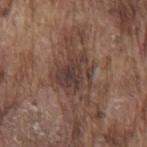Q: Is there a histopathology result?
A: catalogued during a skin exam; not biopsied
Q: Lesion location?
A: the mid back
Q: What did automated image analysis measure?
A: an outline eccentricity of about 0.5 (0 = round, 1 = elongated) and two-axis asymmetry of about 0.3; a nevus-likeness score of about 5/100 and a detector confidence of about 95 out of 100 that the crop contains a lesion
Q: Illumination type?
A: white-light
Q: What are the patient's age and sex?
A: male, approximately 75 years of age
Q: How large is the lesion?
A: ~5 mm (longest diameter)
Q: How was this image acquired?
A: ~15 mm tile from a whole-body skin photo